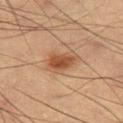follow-up — no biopsy performed (imaged during a skin exam)
image — 15 mm crop, total-body photography
diameter — ≈4 mm
subject — male, in their mid- to late 50s
anatomic site — the right thigh
image-analysis metrics — an average lesion color of about L≈44 a*≈20 b*≈30 (CIELAB), roughly 10 lightness units darker than nearby skin, and a normalized border contrast of about 8; a peripheral color-asymmetry measure near 1; a lesion-detection confidence of about 100/100